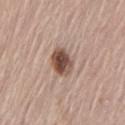Recorded during total-body skin imaging; not selected for excision or biopsy. Located on the leg. A 15 mm close-up tile from a total-body photography series done for melanoma screening. A male subject, aged around 65. This is a white-light tile. About 3.5 mm across.